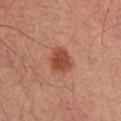{"biopsy_status": "not biopsied; imaged during a skin examination"}